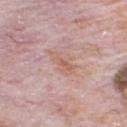Q: Was a biopsy performed?
A: total-body-photography surveillance lesion; no biopsy
Q: Lesion size?
A: about 4 mm
Q: What kind of image is this?
A: 15 mm crop, total-body photography
Q: What are the patient's age and sex?
A: male, approximately 80 years of age
Q: Lesion location?
A: the chest